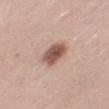Part of a total-body skin-imaging series; this lesion was reviewed on a skin check and was not flagged for biopsy. A female patient, about 30 years old. Automated image analysis of the tile measured a border-irregularity rating of about 1.5/10 and a color-variation rating of about 4.5/10. The analysis additionally found a detector confidence of about 100 out of 100 that the crop contains a lesion. About 4 mm across. Located on the leg. Imaged with white-light lighting. A roughly 15 mm field-of-view crop from a total-body skin photograph.